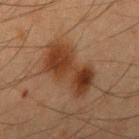Impression:
The lesion was tiled from a total-body skin photograph and was not biopsied.
Image and clinical context:
The recorded lesion diameter is about 7 mm. The lesion is on the left upper arm. A 15 mm close-up tile from a total-body photography series done for melanoma screening. Imaged with cross-polarized lighting. The subject is a male approximately 55 years of age. The lesion-visualizer software estimated a border-irregularity rating of about 3.5/10.